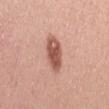workup: total-body-photography surveillance lesion; no biopsy
lighting: white-light
location: the lower back
subject: female, roughly 30 years of age
image-analysis metrics: an eccentricity of roughly 0.85 and a shape-asymmetry score of about 0.2 (0 = symmetric); a lesion color around L≈55 a*≈24 b*≈28 in CIELAB, a lesion–skin lightness drop of about 14, and a normalized lesion–skin contrast near 9.5; a border-irregularity rating of about 2.5/10 and radial color variation of about 1.5; a classifier nevus-likeness of about 90/100
diameter: ~4.5 mm (longest diameter)
image: ~15 mm crop, total-body skin-cancer survey